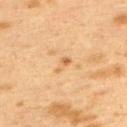Part of a total-body skin-imaging series; this lesion was reviewed on a skin check and was not flagged for biopsy. Longest diameter approximately 2.5 mm. On the upper back. The lesion-visualizer software estimated an average lesion color of about L≈54 a*≈18 b*≈36 (CIELAB), a lesion–skin lightness drop of about 7, and a lesion-to-skin contrast of about 5 (normalized; higher = more distinct). The software also gave an automated nevus-likeness rating near 0 out of 100 and lesion-presence confidence of about 100/100. A lesion tile, about 15 mm wide, cut from a 3D total-body photograph. A female patient, in their 40s.